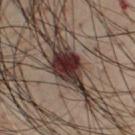Part of a total-body skin-imaging series; this lesion was reviewed on a skin check and was not flagged for biopsy.
The lesion is on the chest.
Approximately 7 mm at its widest.
An algorithmic analysis of the crop reported a lesion color around L≈31 a*≈17 b*≈18 in CIELAB, about 19 CIELAB-L* units darker than the surrounding skin, and a normalized lesion–skin contrast near 16.5.
This is a cross-polarized tile.
A 15 mm close-up tile from a total-body photography series done for melanoma screening.
The subject is a male roughly 55 years of age.